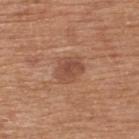notes: imaged on a skin check; not biopsied
automated metrics: a detector confidence of about 100 out of 100 that the crop contains a lesion
diameter: ≈3 mm
image: 15 mm crop, total-body photography
body site: the upper back
subject: male, aged 63 to 67